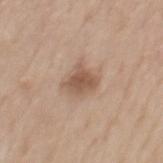Assessment: Recorded during total-body skin imaging; not selected for excision or biopsy. Clinical summary: A male subject, in their mid-60s. Automated tile analysis of the lesion measured a lesion area of about 6.5 mm², an outline eccentricity of about 0.4 (0 = round, 1 = elongated), and a symmetry-axis asymmetry near 0.35. The analysis additionally found a nevus-likeness score of about 65/100 and a lesion-detection confidence of about 100/100. Measured at roughly 3 mm in maximum diameter. This is a white-light tile. The lesion is on the mid back. Cropped from a total-body skin-imaging series; the visible field is about 15 mm.